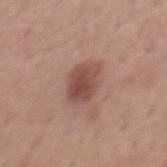The lesion was photographed on a routine skin check and not biopsied; there is no pathology result.